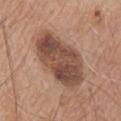Captured during whole-body skin photography for melanoma surveillance; the lesion was not biopsied.
A 15 mm close-up extracted from a 3D total-body photography capture.
From the chest.
A male patient, aged approximately 65.
Captured under white-light illumination.
The lesion-visualizer software estimated an average lesion color of about L≈48 a*≈19 b*≈27 (CIELAB) and a lesion-to-skin contrast of about 10 (normalized; higher = more distinct). It also reported lesion-presence confidence of about 100/100.
Longest diameter approximately 8 mm.